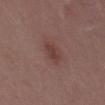– notes: total-body-photography surveillance lesion; no biopsy
– illumination: white-light illumination
– imaging modality: ~15 mm tile from a whole-body skin photo
– subject: male, approximately 50 years of age
– location: the back
– automated metrics: about 7 CIELAB-L* units darker than the surrounding skin and a normalized lesion–skin contrast near 6.5; border irregularity of about 3 on a 0–10 scale, internal color variation of about 1.5 on a 0–10 scale, and peripheral color asymmetry of about 0.5
– lesion diameter: about 3.5 mm